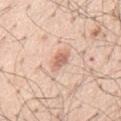Approximately 3 mm at its widest. A male patient, aged 53 to 57. The lesion is on the mid back. A region of skin cropped from a whole-body photographic capture, roughly 15 mm wide.